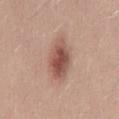follow-up: total-body-photography surveillance lesion; no biopsy
illumination: white-light illumination
image-analysis metrics: an eccentricity of roughly 0.85 and a symmetry-axis asymmetry near 0.2; a nevus-likeness score of about 90/100 and lesion-presence confidence of about 100/100
image source: ~15 mm tile from a whole-body skin photo
body site: the mid back
size: about 6 mm
patient: male, aged around 25